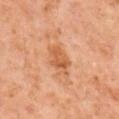The lesion was photographed on a routine skin check and not biopsied; there is no pathology result.
A male patient aged approximately 60.
Captured under cross-polarized illumination.
The total-body-photography lesion software estimated a footprint of about 7 mm², an eccentricity of roughly 0.7, and a symmetry-axis asymmetry near 0.3. And it measured a lesion-to-skin contrast of about 7 (normalized; higher = more distinct).
A 15 mm close-up tile from a total-body photography series done for melanoma screening.
On the mid back.